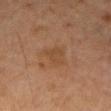| field | value |
|---|---|
| image | total-body-photography crop, ~15 mm field of view |
| lesion diameter | ~3.5 mm (longest diameter) |
| location | the right forearm |
| illumination | cross-polarized |
| patient | female, approximately 70 years of age |